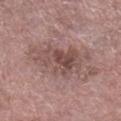{"biopsy_status": "not biopsied; imaged during a skin examination", "image": {"source": "total-body photography crop", "field_of_view_mm": 15}, "site": "right lower leg", "patient": {"sex": "male", "age_approx": 75}}